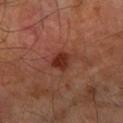Impression:
This lesion was catalogued during total-body skin photography and was not selected for biopsy.
Clinical summary:
Cropped from a whole-body photographic skin survey; the tile spans about 15 mm. From the right forearm. The lesion's longest dimension is about 3 mm. Captured under cross-polarized illumination. A male subject, aged 63 to 67.